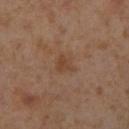{"biopsy_status": "not biopsied; imaged during a skin examination", "site": "right lower leg", "image": {"source": "total-body photography crop", "field_of_view_mm": 15}, "lesion_size": {"long_diameter_mm_approx": 2.5}, "patient": {"sex": "female", "age_approx": 55}, "lighting": "cross-polarized"}